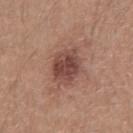biopsy_status: not biopsied; imaged during a skin examination
lesion_size:
  long_diameter_mm_approx: 4.5
site: abdomen
image:
  source: total-body photography crop
  field_of_view_mm: 15
patient:
  sex: male
  age_approx: 20